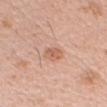Q: Was a biopsy performed?
A: catalogued during a skin exam; not biopsied
Q: How was this image acquired?
A: ~15 mm tile from a whole-body skin photo
Q: Who is the patient?
A: male, aged 33 to 37
Q: Where on the body is the lesion?
A: the head or neck
Q: How was the tile lit?
A: white-light